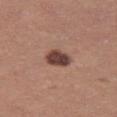The lesion's longest dimension is about 3 mm.
On the leg.
Imaged with white-light lighting.
An algorithmic analysis of the crop reported an area of roughly 6 mm², an eccentricity of roughly 0.6, and a shape-asymmetry score of about 0.2 (0 = symmetric). The software also gave a border-irregularity index near 1.5/10, a color-variation rating of about 3.5/10, and a peripheral color-asymmetry measure near 1.5. The software also gave a nevus-likeness score of about 65/100 and lesion-presence confidence of about 100/100.
A female patient, about 35 years old.
Cropped from a total-body skin-imaging series; the visible field is about 15 mm.